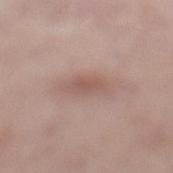Captured during whole-body skin photography for melanoma surveillance; the lesion was not biopsied. This is a white-light tile. The total-body-photography lesion software estimated an average lesion color of about L≈56 a*≈18 b*≈24 (CIELAB), about 8 CIELAB-L* units darker than the surrounding skin, and a lesion-to-skin contrast of about 5.5 (normalized; higher = more distinct). It also reported a nevus-likeness score of about 15/100 and a detector confidence of about 100 out of 100 that the crop contains a lesion. A female patient in their mid-60s. The lesion's longest dimension is about 4.5 mm. A 15 mm close-up extracted from a 3D total-body photography capture. On the leg.